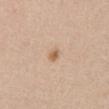* workup · no biopsy performed (imaged during a skin exam)
* patient · female, aged 43 to 47
* site · the abdomen
* tile lighting · white-light
* automated lesion analysis · a nevus-likeness score of about 75/100 and lesion-presence confidence of about 100/100
* image · ~15 mm crop, total-body skin-cancer survey
* size · ≈2 mm